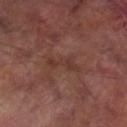| key | value |
|---|---|
| notes | no biopsy performed (imaged during a skin exam) |
| size | ≈4 mm |
| location | the right lower leg |
| automated metrics | a border-irregularity rating of about 6.5/10, a color-variation rating of about 1/10, and radial color variation of about 0 |
| subject | male, roughly 70 years of age |
| lighting | cross-polarized |
| acquisition | ~15 mm crop, total-body skin-cancer survey |